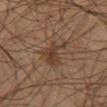Q: Was this lesion biopsied?
A: imaged on a skin check; not biopsied
Q: Where on the body is the lesion?
A: the right thigh
Q: What did automated image analysis measure?
A: an average lesion color of about L≈35 a*≈15 b*≈25 (CIELAB) and roughly 7 lightness units darker than nearby skin; a nevus-likeness score of about 15/100 and lesion-presence confidence of about 100/100
Q: Illumination type?
A: cross-polarized illumination
Q: What are the patient's age and sex?
A: male, in their mid- to late 50s
Q: How was this image acquired?
A: total-body-photography crop, ~15 mm field of view
Q: How large is the lesion?
A: about 5 mm